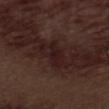Assessment:
The lesion was tiled from a total-body skin photograph and was not biopsied.
Context:
This is a white-light tile. A close-up tile cropped from a whole-body skin photograph, about 15 mm across. The patient is a male roughly 70 years of age. The lesion is on the abdomen. Measured at roughly 3.5 mm in maximum diameter.